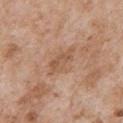<lesion>
<biopsy_status>not biopsied; imaged during a skin examination</biopsy_status>
<lighting>white-light</lighting>
<image>
  <source>total-body photography crop</source>
  <field_of_view_mm>15</field_of_view_mm>
</image>
<patient>
  <sex>male</sex>
  <age_approx>65</age_approx>
</patient>
<automated_metrics>
  <cielab_L>55</cielab_L>
  <cielab_a>20</cielab_a>
  <cielab_b>32</cielab_b>
  <vs_skin_darker_L>8.0</vs_skin_darker_L>
  <vs_skin_contrast_norm>5.5</vs_skin_contrast_norm>
  <border_irregularity_0_10>4.0</border_irregularity_0_10>
  <color_variation_0_10>2.5</color_variation_0_10>
  <peripheral_color_asymmetry>1.0</peripheral_color_asymmetry>
</automated_metrics>
<lesion_size>
  <long_diameter_mm_approx>4.0</long_diameter_mm_approx>
</lesion_size>
<site>front of the torso</site>
</lesion>